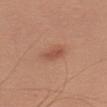biopsy status = no biopsy performed (imaged during a skin exam)
automated metrics = border irregularity of about 2.5 on a 0–10 scale and peripheral color asymmetry of about 0.5
patient = male, aged approximately 45
lesion diameter = about 3 mm
illumination = white-light illumination
imaging modality = 15 mm crop, total-body photography
site = the left upper arm